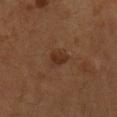On the chest. A male patient aged 53–57. A roughly 15 mm field-of-view crop from a total-body skin photograph.